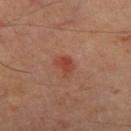Impression:
Part of a total-body skin-imaging series; this lesion was reviewed on a skin check and was not flagged for biopsy.
Context:
A male subject, in their mid-60s. A 15 mm crop from a total-body photograph taken for skin-cancer surveillance. On the leg. The tile uses cross-polarized illumination. The lesion's longest dimension is about 2.5 mm.